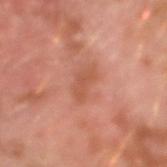The lesion was photographed on a routine skin check and not biopsied; there is no pathology result. A region of skin cropped from a whole-body photographic capture, roughly 15 mm wide. On the left forearm. This is a cross-polarized tile. The subject is a male roughly 30 years of age.